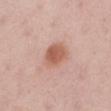This lesion was catalogued during total-body skin photography and was not selected for biopsy. Measured at roughly 3.5 mm in maximum diameter. The lesion is on the left lower leg. A female subject, approximately 45 years of age. Automated image analysis of the tile measured a color-variation rating of about 3/10 and peripheral color asymmetry of about 1. The analysis additionally found a classifier nevus-likeness of about 100/100 and lesion-presence confidence of about 100/100. A region of skin cropped from a whole-body photographic capture, roughly 15 mm wide.